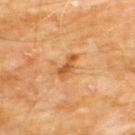notes: total-body-photography surveillance lesion; no biopsy | illumination: cross-polarized | automated metrics: an area of roughly 4 mm² and a shape eccentricity near 0.9 | image source: ~15 mm tile from a whole-body skin photo | diameter: about 3 mm | body site: the chest | patient: male, approximately 60 years of age.